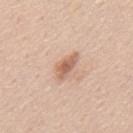follow-up — no biopsy performed (imaged during a skin exam); TBP lesion metrics — an average lesion color of about L≈62 a*≈21 b*≈31 (CIELAB), roughly 12 lightness units darker than nearby skin, and a normalized lesion–skin contrast near 7.5; diameter — about 3.5 mm; image source — ~15 mm crop, total-body skin-cancer survey; anatomic site — the mid back; subject — male, in their mid-40s.